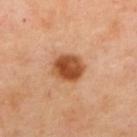Q: Was this lesion biopsied?
A: imaged on a skin check; not biopsied
Q: Patient demographics?
A: female, aged 38 to 42
Q: How was this image acquired?
A: ~15 mm tile from a whole-body skin photo
Q: Lesion location?
A: the back